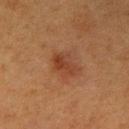Impression: The lesion was tiled from a total-body skin photograph and was not biopsied. Acquisition and patient details: This is a cross-polarized tile. Cropped from a total-body skin-imaging series; the visible field is about 15 mm. A female patient approximately 55 years of age. From the right upper arm.